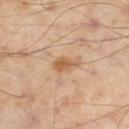Assessment: Part of a total-body skin-imaging series; this lesion was reviewed on a skin check and was not flagged for biopsy. Background: A 15 mm crop from a total-body photograph taken for skin-cancer surveillance. A male patient, aged approximately 55. The lesion's longest dimension is about 3 mm. Captured under cross-polarized illumination. Located on the left thigh.